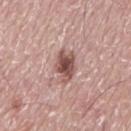workup — total-body-photography surveillance lesion; no biopsy
image-analysis metrics — a footprint of about 7.5 mm², a shape eccentricity near 0.8, and two-axis asymmetry of about 0.3; a mean CIELAB color near L≈51 a*≈21 b*≈23 and a lesion–skin lightness drop of about 15; border irregularity of about 3.5 on a 0–10 scale, internal color variation of about 4.5 on a 0–10 scale, and radial color variation of about 1
patient — male, aged 73 to 77
lighting — white-light
acquisition — total-body-photography crop, ~15 mm field of view
body site — the mid back
size — ~4 mm (longest diameter)